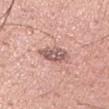workup — no biopsy performed (imaged during a skin exam)
illumination — white-light illumination
automated metrics — a lesion area of about 7.5 mm², a shape eccentricity near 0.8, and a symmetry-axis asymmetry near 0.2; an automated nevus-likeness rating near 0 out of 100
image — 15 mm crop, total-body photography
size — ~4 mm (longest diameter)
location — the left upper arm
subject — male, in their mid- to late 30s